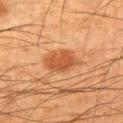workup: total-body-photography surveillance lesion; no biopsy | subject: male, aged 53–57 | lesion diameter: about 4 mm | acquisition: ~15 mm crop, total-body skin-cancer survey | location: the right upper arm | automated metrics: an average lesion color of about L≈47 a*≈25 b*≈37 (CIELAB), about 10 CIELAB-L* units darker than the surrounding skin, and a normalized border contrast of about 7.5; border irregularity of about 2 on a 0–10 scale and peripheral color asymmetry of about 0.5; a classifier nevus-likeness of about 95/100 and a lesion-detection confidence of about 100/100 | tile lighting: cross-polarized illumination.